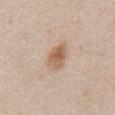{"automated_metrics": {"area_mm2_approx": 6.5, "eccentricity": 0.65}, "image": {"source": "total-body photography crop", "field_of_view_mm": 15}, "lesion_size": {"long_diameter_mm_approx": 3.0}, "patient": {"sex": "female", "age_approx": 60}, "site": "abdomen", "lighting": "white-light"}